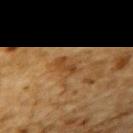biopsy status: no biopsy performed (imaged during a skin exam); tile lighting: cross-polarized illumination; subject: male, about 85 years old or older; location: the upper back; acquisition: ~15 mm tile from a whole-body skin photo; lesion diameter: ~2.5 mm (longest diameter).